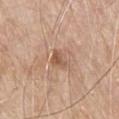Notes:
• biopsy status · catalogued during a skin exam; not biopsied
• illumination · white-light illumination
• subject · male, approximately 80 years of age
• site · the chest
• image source · total-body-photography crop, ~15 mm field of view